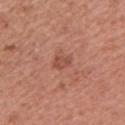Impression:
Part of a total-body skin-imaging series; this lesion was reviewed on a skin check and was not flagged for biopsy.
Background:
The lesion's longest dimension is about 2.5 mm. Cropped from a total-body skin-imaging series; the visible field is about 15 mm. The patient is a male in their mid-40s. The total-body-photography lesion software estimated an outline eccentricity of about 0.8 (0 = round, 1 = elongated). And it measured a border-irregularity rating of about 3/10, a color-variation rating of about 1.5/10, and a peripheral color-asymmetry measure near 0.5. And it measured lesion-presence confidence of about 100/100. The lesion is located on the arm. Captured under white-light illumination.